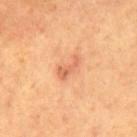Part of a total-body skin-imaging series; this lesion was reviewed on a skin check and was not flagged for biopsy. A lesion tile, about 15 mm wide, cut from a 3D total-body photograph. The subject is a male aged approximately 55. The lesion's longest dimension is about 3.5 mm. The lesion is on the mid back.